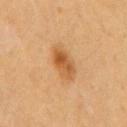Captured during whole-body skin photography for melanoma surveillance; the lesion was not biopsied.
Approximately 4.5 mm at its widest.
This is a cross-polarized tile.
A male subject, aged approximately 60.
Automated image analysis of the tile measured an area of roughly 8 mm², a shape eccentricity near 0.85, and a symmetry-axis asymmetry near 0.15. The analysis additionally found an average lesion color of about L≈53 a*≈22 b*≈41 (CIELAB) and roughly 11 lightness units darker than nearby skin. The software also gave an automated nevus-likeness rating near 85 out of 100 and a lesion-detection confidence of about 100/100.
On the chest.
A close-up tile cropped from a whole-body skin photograph, about 15 mm across.